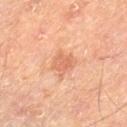No biopsy was performed on this lesion — it was imaged during a full skin examination and was not determined to be concerning. The total-body-photography lesion software estimated an area of roughly 6.5 mm². It also reported a border-irregularity rating of about 3.5/10, a color-variation rating of about 2.5/10, and radial color variation of about 1. A male subject approximately 65 years of age. A 15 mm close-up extracted from a 3D total-body photography capture. Captured under cross-polarized illumination. The lesion is located on the left thigh.